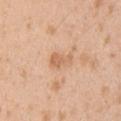notes=no biopsy performed (imaged during a skin exam) | diameter=≈3 mm | subject=male, aged around 25 | lighting=white-light | image source=total-body-photography crop, ~15 mm field of view | anatomic site=the right upper arm.